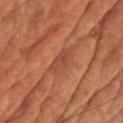Clinical impression: This lesion was catalogued during total-body skin photography and was not selected for biopsy. Image and clinical context: This image is a 15 mm lesion crop taken from a total-body photograph. The tile uses cross-polarized illumination. The lesion is on the upper back. A male subject, aged around 65.